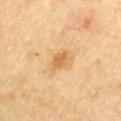| field | value |
|---|---|
| biopsy status | total-body-photography surveillance lesion; no biopsy |
| patient | male, aged around 65 |
| imaging modality | total-body-photography crop, ~15 mm field of view |
| location | the left upper arm |